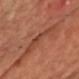Q: Is there a histopathology result?
A: no biopsy performed (imaged during a skin exam)
Q: What did automated image analysis measure?
A: lesion-presence confidence of about 65/100
Q: How was the tile lit?
A: cross-polarized illumination
Q: How was this image acquired?
A: 15 mm crop, total-body photography
Q: What is the anatomic site?
A: the chest
Q: Lesion size?
A: ≈5 mm
Q: Patient demographics?
A: approximately 55 years of age